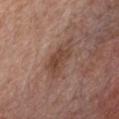Part of a total-body skin-imaging series; this lesion was reviewed on a skin check and was not flagged for biopsy.
The tile uses white-light illumination.
A region of skin cropped from a whole-body photographic capture, roughly 15 mm wide.
The lesion-visualizer software estimated an area of roughly 8.5 mm², an outline eccentricity of about 0.9 (0 = round, 1 = elongated), and two-axis asymmetry of about 0.3. It also reported an average lesion color of about L≈44 a*≈19 b*≈26 (CIELAB). It also reported border irregularity of about 4 on a 0–10 scale, a color-variation rating of about 3.5/10, and radial color variation of about 1.5. It also reported a nevus-likeness score of about 20/100 and lesion-presence confidence of about 100/100.
On the chest.
Approximately 5 mm at its widest.
A male subject roughly 60 years of age.